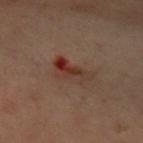A female patient, aged 58–62. An algorithmic analysis of the crop reported a footprint of about 8 mm², a shape eccentricity near 0.9, and a symmetry-axis asymmetry near 0.35. It also reported a lesion color around L≈30 a*≈18 b*≈22 in CIELAB, roughly 7 lightness units darker than nearby skin, and a normalized border contrast of about 8. It also reported a lesion-detection confidence of about 100/100. On the right arm. The lesion's longest dimension is about 4.5 mm. Captured under cross-polarized illumination. A roughly 15 mm field-of-view crop from a total-body skin photograph.